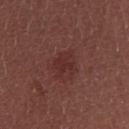follow-up: no biopsy performed (imaged during a skin exam) | body site: the back | lesion diameter: ≈3 mm | patient: female, aged 23–27 | imaging modality: ~15 mm tile from a whole-body skin photo | illumination: white-light illumination | image-analysis metrics: a lesion area of about 6.5 mm², an eccentricity of roughly 0.55, and two-axis asymmetry of about 0.25; a border-irregularity index near 3.5/10 and radial color variation of about 0.5.